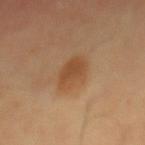notes = imaged on a skin check; not biopsied
site = the mid back
size = about 4 mm
illumination = cross-polarized
TBP lesion metrics = a lesion area of about 8.5 mm², a shape eccentricity near 0.8, and two-axis asymmetry of about 0.2; an automated nevus-likeness rating near 90 out of 100 and a detector confidence of about 100 out of 100 that the crop contains a lesion
patient = male, in their mid- to late 60s
image = 15 mm crop, total-body photography